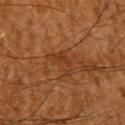{"biopsy_status": "not biopsied; imaged during a skin examination", "image": {"source": "total-body photography crop", "field_of_view_mm": 15}, "patient": {"sex": "male", "age_approx": 60}, "lesion_size": {"long_diameter_mm_approx": 3.5}, "site": "upper back"}